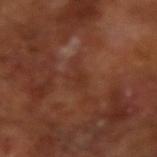Recorded during total-body skin imaging; not selected for excision or biopsy.
A roughly 15 mm field-of-view crop from a total-body skin photograph.
An algorithmic analysis of the crop reported a nevus-likeness score of about 0/100 and lesion-presence confidence of about 95/100.
A male patient, approximately 65 years of age.
Longest diameter approximately 2.5 mm.